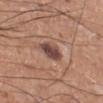lighting: white-light
anatomic site: the right lower leg
lesion size: ≈3 mm
patient: male, approximately 55 years of age
TBP lesion metrics: a shape eccentricity near 0.65 and a shape-asymmetry score of about 0.2 (0 = symmetric); a mean CIELAB color near L≈45 a*≈20 b*≈23 and a lesion-to-skin contrast of about 10.5 (normalized; higher = more distinct); a classifier nevus-likeness of about 15/100 and a detector confidence of about 100 out of 100 that the crop contains a lesion
acquisition: 15 mm crop, total-body photography